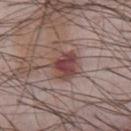- workup — catalogued during a skin exam; not biopsied
- imaging modality — ~15 mm crop, total-body skin-cancer survey
- tile lighting — white-light
- lesion diameter — about 4 mm
- patient — male, aged around 65
- body site — the chest